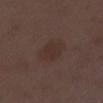biopsy status = imaged on a skin check; not biopsied
lighting = white-light
automated lesion analysis = a mean CIELAB color near L≈30 a*≈15 b*≈20, roughly 5 lightness units darker than nearby skin, and a normalized lesion–skin contrast near 5.5
lesion size = about 3 mm
anatomic site = the right lower leg
patient = female, aged around 50
acquisition = ~15 mm crop, total-body skin-cancer survey